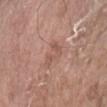Captured during whole-body skin photography for melanoma surveillance; the lesion was not biopsied.
About 3 mm across.
A female subject, aged approximately 75.
The lesion is located on the arm.
This is a white-light tile.
Cropped from a whole-body photographic skin survey; the tile spans about 15 mm.